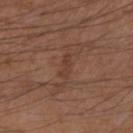* workup: total-body-photography surveillance lesion; no biopsy
* anatomic site: the right upper arm
* acquisition: ~15 mm crop, total-body skin-cancer survey
* diameter: about 3 mm
* subject: male, aged 48 to 52
* TBP lesion metrics: a nevus-likeness score of about 0/100